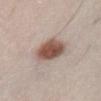Assessment: Imaged during a routine full-body skin examination; the lesion was not biopsied and no histopathology is available. Context: A roughly 15 mm field-of-view crop from a total-body skin photograph. A male patient approximately 25 years of age. The lesion is on the abdomen.